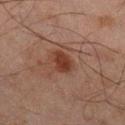  biopsy_status: not biopsied; imaged during a skin examination
  site: right lower leg
  image:
    source: total-body photography crop
    field_of_view_mm: 15
  patient:
    sex: male
    age_approx: 45
  lesion_size:
    long_diameter_mm_approx: 3.0
  lighting: cross-polarized